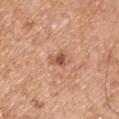anatomic site: the right upper arm
acquisition: total-body-photography crop, ~15 mm field of view
automated lesion analysis: an average lesion color of about L≈54 a*≈24 b*≈32 (CIELAB), a lesion–skin lightness drop of about 11, and a lesion-to-skin contrast of about 7.5 (normalized; higher = more distinct); a classifier nevus-likeness of about 75/100 and a lesion-detection confidence of about 100/100
diameter: ~2.5 mm (longest diameter)
subject: male, aged around 55
lighting: white-light illumination